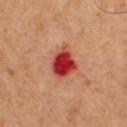This lesion was catalogued during total-body skin photography and was not selected for biopsy. The tile uses cross-polarized illumination. A male subject, approximately 50 years of age. A 15 mm close-up tile from a total-body photography series done for melanoma screening. An algorithmic analysis of the crop reported an automated nevus-likeness rating near 0 out of 100 and a detector confidence of about 100 out of 100 that the crop contains a lesion. The lesion is on the front of the torso.